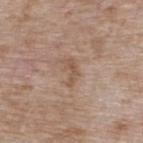{
  "biopsy_status": "not biopsied; imaged during a skin examination",
  "image": {
    "source": "total-body photography crop",
    "field_of_view_mm": 15
  },
  "lighting": "white-light",
  "patient": {
    "sex": "female",
    "age_approx": 75
  },
  "lesion_size": {
    "long_diameter_mm_approx": 3.0
  },
  "site": "upper back"
}